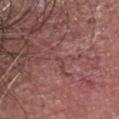A 15 mm close-up extracted from a 3D total-body photography capture. The lesion is located on the head or neck. Automated image analysis of the tile measured a footprint of about 0.5 mm², a shape eccentricity near 0.7, and a shape-asymmetry score of about 0.35 (0 = symmetric). The software also gave a border-irregularity index near 2.5/10 and a color-variation rating of about 0/10. The analysis additionally found a classifier nevus-likeness of about 0/100 and lesion-presence confidence of about 0/100. The tile uses white-light illumination. The patient is a male aged approximately 45. Histopathologically confirmed as a superficial basal cell carcinoma (malignant).